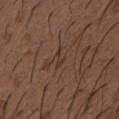| field | value |
|---|---|
| notes | imaged on a skin check; not biopsied |
| patient | male, about 50 years old |
| anatomic site | the chest |
| automated metrics | a border-irregularity index near 6/10 and a peripheral color-asymmetry measure near 0; a nevus-likeness score of about 0/100 and a detector confidence of about 65 out of 100 that the crop contains a lesion |
| lesion diameter | ≈3 mm |
| image | total-body-photography crop, ~15 mm field of view |
| illumination | white-light illumination |